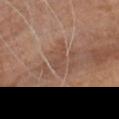The lesion was tiled from a total-body skin photograph and was not biopsied.
A lesion tile, about 15 mm wide, cut from a 3D total-body photograph.
The subject is a male about 70 years old.
Captured under cross-polarized illumination.
From the head or neck.
About 4.5 mm across.